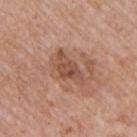follow-up — no biopsy performed (imaged during a skin exam)
anatomic site — the left upper arm
subject — male, aged 73 to 77
automated metrics — a footprint of about 8 mm², an eccentricity of roughly 0.85, and a shape-asymmetry score of about 0.4 (0 = symmetric); border irregularity of about 4.5 on a 0–10 scale and a peripheral color-asymmetry measure near 1.5
acquisition — 15 mm crop, total-body photography
lighting — white-light illumination
size — about 5 mm